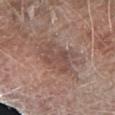Captured during whole-body skin photography for melanoma surveillance; the lesion was not biopsied. A close-up tile cropped from a whole-body skin photograph, about 15 mm across. On the arm. A male patient aged approximately 80.